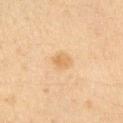Notes:
* biopsy status: no biopsy performed (imaged during a skin exam)
* size: ~3 mm (longest diameter)
* tile lighting: cross-polarized illumination
* patient: female, approximately 40 years of age
* site: the right upper arm
* acquisition: 15 mm crop, total-body photography
* TBP lesion metrics: an area of roughly 5 mm², a shape eccentricity near 0.55, and a shape-asymmetry score of about 0.2 (0 = symmetric); a border-irregularity index near 2/10, internal color variation of about 2 on a 0–10 scale, and radial color variation of about 0.5; lesion-presence confidence of about 100/100